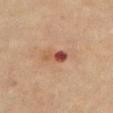biopsy status: catalogued during a skin exam; not biopsied
patient: female, aged 78–82
site: the chest
acquisition: 15 mm crop, total-body photography